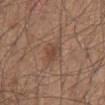biopsy status: imaged on a skin check; not biopsied | tile lighting: white-light | location: the abdomen | image source: ~15 mm tile from a whole-body skin photo | subject: male, about 65 years old.